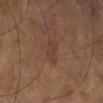Q: Was a biopsy performed?
A: imaged on a skin check; not biopsied
Q: Where on the body is the lesion?
A: the left leg
Q: What is the imaging modality?
A: ~15 mm crop, total-body skin-cancer survey
Q: What lighting was used for the tile?
A: cross-polarized illumination
Q: What did automated image analysis measure?
A: a mean CIELAB color near L≈37 a*≈18 b*≈25, about 5 CIELAB-L* units darker than the surrounding skin, and a lesion-to-skin contrast of about 4.5 (normalized; higher = more distinct); a within-lesion color-variation index near 1/10 and a peripheral color-asymmetry measure near 0.5; an automated nevus-likeness rating near 0 out of 100 and lesion-presence confidence of about 100/100
Q: Who is the patient?
A: male, aged 63 to 67